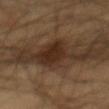| key | value |
|---|---|
| biopsy status | no biopsy performed (imaged during a skin exam) |
| lesion diameter | ≈7.5 mm |
| site | the abdomen |
| imaging modality | ~15 mm tile from a whole-body skin photo |
| tile lighting | cross-polarized illumination |
| patient | male, approximately 60 years of age |